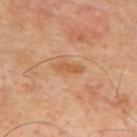Impression: The lesion was photographed on a routine skin check and not biopsied; there is no pathology result. Context: The lesion's longest dimension is about 3 mm. A 15 mm close-up extracted from a 3D total-body photography capture. The lesion is on the upper back. The tile uses cross-polarized illumination. The patient is a male aged 63–67.